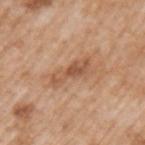Q: Is there a histopathology result?
A: total-body-photography surveillance lesion; no biopsy
Q: How was this image acquired?
A: ~15 mm crop, total-body skin-cancer survey
Q: Lesion size?
A: ≈4.5 mm
Q: Illumination type?
A: white-light
Q: Automated lesion metrics?
A: about 10 CIELAB-L* units darker than the surrounding skin; a border-irregularity rating of about 4/10, a within-lesion color-variation index near 4/10, and a peripheral color-asymmetry measure near 1; a classifier nevus-likeness of about 0/100 and a lesion-detection confidence of about 100/100
Q: Where on the body is the lesion?
A: the left upper arm
Q: Who is the patient?
A: male, aged 63–67